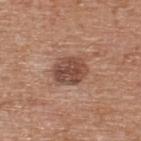follow-up: total-body-photography surveillance lesion; no biopsy | subject: male, roughly 65 years of age | site: the upper back | automated lesion analysis: a lesion area of about 10 mm², a shape eccentricity near 0.6, and two-axis asymmetry of about 0.1; a lesion color around L≈47 a*≈21 b*≈27 in CIELAB, about 12 CIELAB-L* units darker than the surrounding skin, and a normalized lesion–skin contrast near 9; border irregularity of about 1 on a 0–10 scale, internal color variation of about 4.5 on a 0–10 scale, and radial color variation of about 1.5 | acquisition: total-body-photography crop, ~15 mm field of view | size: ~4 mm (longest diameter) | tile lighting: white-light.